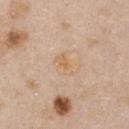| feature | finding |
|---|---|
| biopsy status | imaged on a skin check; not biopsied |
| tile lighting | white-light |
| image | ~15 mm crop, total-body skin-cancer survey |
| patient | female, roughly 45 years of age |
| automated lesion analysis | an average lesion color of about L≈65 a*≈17 b*≈36 (CIELAB), about 5 CIELAB-L* units darker than the surrounding skin, and a normalized lesion–skin contrast near 6; border irregularity of about 4 on a 0–10 scale, a within-lesion color-variation index near 1.5/10, and radial color variation of about 0.5; a classifier nevus-likeness of about 0/100 and a detector confidence of about 100 out of 100 that the crop contains a lesion |
| lesion size | ≈3 mm |
| body site | the chest |